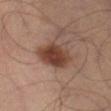Impression: Recorded during total-body skin imaging; not selected for excision or biopsy. Acquisition and patient details: A lesion tile, about 15 mm wide, cut from a 3D total-body photograph. Located on the leg. A male patient aged around 65.